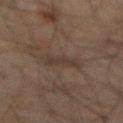notes=no biopsy performed (imaged during a skin exam) | lighting=cross-polarized illumination | diameter=about 3.5 mm | body site=the abdomen | patient=male, aged approximately 60 | image=~15 mm tile from a whole-body skin photo.